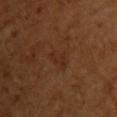Acquisition and patient details: The subject is a female aged 53–57. Cropped from a whole-body photographic skin survey; the tile spans about 15 mm. Imaged with cross-polarized lighting. From the chest. Longest diameter approximately 2.5 mm.